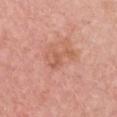- follow-up · no biopsy performed (imaged during a skin exam)
- image · 15 mm crop, total-body photography
- subject · female, in their 40s
- anatomic site · the chest